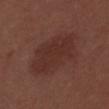The lesion was tiled from a total-body skin photograph and was not biopsied. A male patient, in their 30s. Cropped from a whole-body photographic skin survey; the tile spans about 15 mm. On the mid back.